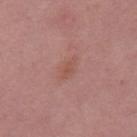| feature | finding |
|---|---|
| notes | total-body-photography surveillance lesion; no biopsy |
| patient | female, aged 38–42 |
| acquisition | total-body-photography crop, ~15 mm field of view |
| lesion diameter | ≈3 mm |
| lighting | white-light |
| location | the right thigh |